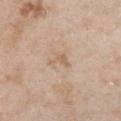notes = catalogued during a skin exam; not biopsied
automated metrics = a footprint of about 3 mm², an eccentricity of roughly 0.75, and two-axis asymmetry of about 0.6; a mean CIELAB color near L≈62 a*≈16 b*≈32 and a lesion–skin lightness drop of about 7
subject = female, aged around 30
size = ~2.5 mm (longest diameter)
lighting = white-light illumination
image source = ~15 mm tile from a whole-body skin photo
site = the front of the torso